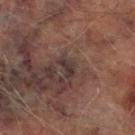Clinical impression: No biopsy was performed on this lesion — it was imaged during a full skin examination and was not determined to be concerning. Background: A roughly 15 mm field-of-view crop from a total-body skin photograph. A male patient aged around 75. The lesion is located on the right lower leg. Measured at roughly 2.5 mm in maximum diameter.